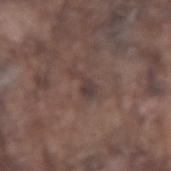Recorded during total-body skin imaging; not selected for excision or biopsy.
Cropped from a total-body skin-imaging series; the visible field is about 15 mm.
An algorithmic analysis of the crop reported a normalized lesion–skin contrast near 6.5.
The patient is a male in their mid-70s.
The tile uses white-light illumination.
On the right forearm.